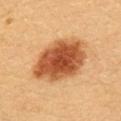Assessment:
Captured during whole-body skin photography for melanoma surveillance; the lesion was not biopsied.
Background:
The subject is a female roughly 30 years of age. A 15 mm close-up extracted from a 3D total-body photography capture. Located on the upper back.